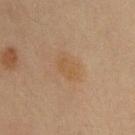Background:
The tile uses cross-polarized illumination. The recorded lesion diameter is about 3.5 mm. The total-body-photography lesion software estimated a border-irregularity index near 2.5/10 and a color-variation rating of about 2/10. The software also gave a nevus-likeness score of about 0/100. A 15 mm close-up extracted from a 3D total-body photography capture. A male patient, aged 33–37. On the upper back.